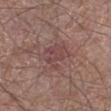{"site": "leg", "image": {"source": "total-body photography crop", "field_of_view_mm": 15}, "patient": {"sex": "male", "age_approx": 60}}